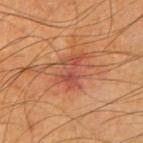Part of a total-body skin-imaging series; this lesion was reviewed on a skin check and was not flagged for biopsy. This is a cross-polarized tile. A male subject, roughly 65 years of age. The lesion-visualizer software estimated a mean CIELAB color near L≈51 a*≈30 b*≈33. The analysis additionally found a border-irregularity rating of about 6/10, a color-variation rating of about 5.5/10, and a peripheral color-asymmetry measure near 2. And it measured a classifier nevus-likeness of about 0/100. A 15 mm crop from a total-body photograph taken for skin-cancer surveillance. The lesion is on the right upper arm. Longest diameter approximately 4 mm.